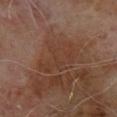Measured at roughly 6.5 mm in maximum diameter. On the upper back. A roughly 15 mm field-of-view crop from a total-body skin photograph. A male subject roughly 65 years of age.